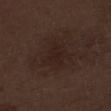<lesion>
<biopsy_status>not biopsied; imaged during a skin examination</biopsy_status>
<site>left lower leg</site>
<image>
  <source>total-body photography crop</source>
  <field_of_view_mm>15</field_of_view_mm>
</image>
<patient>
  <sex>male</sex>
  <age_approx>70</age_approx>
</patient>
</lesion>